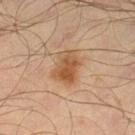{"biopsy_status": "not biopsied; imaged during a skin examination", "patient": {"sex": "male", "age_approx": 65}, "lesion_size": {"long_diameter_mm_approx": 4.0}, "image": {"source": "total-body photography crop", "field_of_view_mm": 15}, "lighting": "cross-polarized", "site": "leg"}